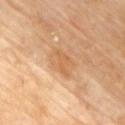Imaged during a routine full-body skin examination; the lesion was not biopsied and no histopathology is available. From the chest. A 15 mm crop from a total-body photograph taken for skin-cancer surveillance. Captured under cross-polarized illumination. About 4 mm across. The total-body-photography lesion software estimated an area of roughly 7 mm², an eccentricity of roughly 0.8, and a symmetry-axis asymmetry near 0.25. It also reported internal color variation of about 1.5 on a 0–10 scale and a peripheral color-asymmetry measure near 0.5. And it measured an automated nevus-likeness rating near 5 out of 100. The subject is a male aged approximately 70.